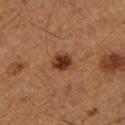Recorded during total-body skin imaging; not selected for excision or biopsy. The patient is a male roughly 75 years of age. This is a cross-polarized tile. Automated image analysis of the tile measured an area of roughly 5 mm², an outline eccentricity of about 0.55 (0 = round, 1 = elongated), and two-axis asymmetry of about 0.15. And it measured an average lesion color of about L≈27 a*≈19 b*≈25 (CIELAB), roughly 11 lightness units darker than nearby skin, and a normalized border contrast of about 11. The analysis additionally found a border-irregularity rating of about 1.5/10, a color-variation rating of about 2.5/10, and peripheral color asymmetry of about 0.5. The analysis additionally found a classifier nevus-likeness of about 100/100. On the left lower leg. This image is a 15 mm lesion crop taken from a total-body photograph. About 2.5 mm across.